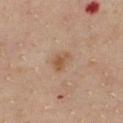Q: Was this lesion biopsied?
A: total-body-photography surveillance lesion; no biopsy
Q: Lesion location?
A: the chest
Q: Patient demographics?
A: female, approximately 45 years of age
Q: Illumination type?
A: cross-polarized illumination
Q: What did automated image analysis measure?
A: a lesion area of about 4.5 mm², an eccentricity of roughly 0.7, and a shape-asymmetry score of about 0.35 (0 = symmetric); a nevus-likeness score of about 55/100 and lesion-presence confidence of about 100/100
Q: What is the lesion's diameter?
A: ~2.5 mm (longest diameter)
Q: What kind of image is this?
A: ~15 mm tile from a whole-body skin photo